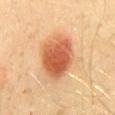Notes:
- follow-up · catalogued during a skin exam; not biopsied
- lesion diameter · ~5.5 mm (longest diameter)
- illumination · cross-polarized
- subject · male, roughly 30 years of age
- anatomic site · the mid back
- imaging modality · total-body-photography crop, ~15 mm field of view